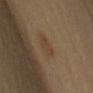The lesion was tiled from a total-body skin photograph and was not biopsied. A close-up tile cropped from a whole-body skin photograph, about 15 mm across. A female subject, aged around 45. From the left upper arm. Longest diameter approximately 3 mm.